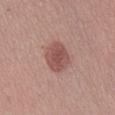The lesion was photographed on a routine skin check and not biopsied; there is no pathology result.
A female patient, in their mid- to late 30s.
A 15 mm close-up tile from a total-body photography series done for melanoma screening.
On the abdomen.
The recorded lesion diameter is about 4 mm.